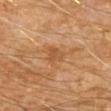This lesion was catalogued during total-body skin photography and was not selected for biopsy.
A male patient, approximately 60 years of age.
Cropped from a total-body skin-imaging series; the visible field is about 15 mm.
The lesion is on the right upper arm.